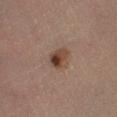{"biopsy_status": "not biopsied; imaged during a skin examination", "lighting": "cross-polarized", "lesion_size": {"long_diameter_mm_approx": 3.0}, "site": "left lower leg", "image": {"source": "total-body photography crop", "field_of_view_mm": 15}, "patient": {"sex": "female", "age_approx": 60}}